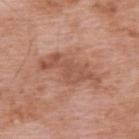anatomic site — the upper back
imaging modality — ~15 mm crop, total-body skin-cancer survey
patient — male, roughly 60 years of age